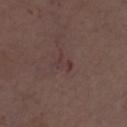{
  "biopsy_status": "not biopsied; imaged during a skin examination",
  "site": "right upper arm",
  "patient": {
    "sex": "male",
    "age_approx": 70
  },
  "image": {
    "source": "total-body photography crop",
    "field_of_view_mm": 15
  },
  "lighting": "white-light"
}